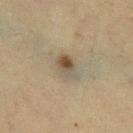Clinical impression: The lesion was photographed on a routine skin check and not biopsied; there is no pathology result. Acquisition and patient details: An algorithmic analysis of the crop reported a footprint of about 5.5 mm², an outline eccentricity of about 0.75 (0 = round, 1 = elongated), and a symmetry-axis asymmetry near 0.2. The software also gave a border-irregularity index near 2/10 and peripheral color asymmetry of about 2.5. Approximately 3 mm at its widest. Imaged with cross-polarized lighting. This image is a 15 mm lesion crop taken from a total-body photograph. The subject is a female in their mid-50s. On the leg.